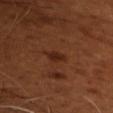Recorded during total-body skin imaging; not selected for excision or biopsy.